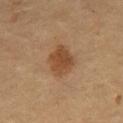{"biopsy_status": "not biopsied; imaged during a skin examination", "lesion_size": {"long_diameter_mm_approx": 4.5}, "site": "abdomen", "image": {"source": "total-body photography crop", "field_of_view_mm": 15}, "patient": {"sex": "male", "age_approx": 55}, "lighting": "cross-polarized"}